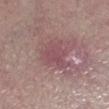tile lighting: white-light illumination | site: the left lower leg | subject: female, roughly 65 years of age | image source: ~15 mm tile from a whole-body skin photo.